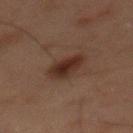Q: Is there a histopathology result?
A: no biopsy performed (imaged during a skin exam)
Q: How large is the lesion?
A: ≈4.5 mm
Q: What is the imaging modality?
A: total-body-photography crop, ~15 mm field of view
Q: What is the anatomic site?
A: the back
Q: How was the tile lit?
A: cross-polarized illumination
Q: What are the patient's age and sex?
A: male, approximately 60 years of age